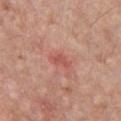{"biopsy_status": "not biopsied; imaged during a skin examination", "image": {"source": "total-body photography crop", "field_of_view_mm": 15}, "lesion_size": {"long_diameter_mm_approx": 3.0}, "automated_metrics": {"area_mm2_approx": 3.5, "eccentricity": 0.85, "shape_asymmetry": 0.3, "vs_skin_darker_L": 8.0, "nevus_likeness_0_100": 0, "lesion_detection_confidence_0_100": 100}, "patient": {"sex": "male", "age_approx": 60}, "site": "chest", "lighting": "white-light"}